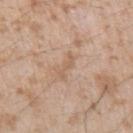notes — imaged on a skin check; not biopsied | patient — male, roughly 25 years of age | imaging modality — total-body-photography crop, ~15 mm field of view | location — the left upper arm.